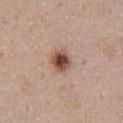Q: What are the patient's age and sex?
A: female, about 50 years old
Q: What did automated image analysis measure?
A: border irregularity of about 2 on a 0–10 scale, internal color variation of about 8 on a 0–10 scale, and peripheral color asymmetry of about 2; an automated nevus-likeness rating near 100 out of 100 and a lesion-detection confidence of about 100/100
Q: How was this image acquired?
A: total-body-photography crop, ~15 mm field of view
Q: What is the anatomic site?
A: the back
Q: Lesion size?
A: ~3 mm (longest diameter)
Q: Illumination type?
A: white-light illumination